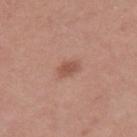The total-body-photography lesion software estimated an area of roughly 3.5 mm², an outline eccentricity of about 0.8 (0 = round, 1 = elongated), and two-axis asymmetry of about 0.2. The analysis additionally found roughly 9 lightness units darker than nearby skin and a normalized border contrast of about 7. And it measured a border-irregularity rating of about 2/10, a within-lesion color-variation index near 1.5/10, and a peripheral color-asymmetry measure near 0.5. It also reported a nevus-likeness score of about 85/100 and a lesion-detection confidence of about 100/100.
A female patient in their mid- to late 40s.
Cropped from a whole-body photographic skin survey; the tile spans about 15 mm.
Imaged with white-light lighting.
The lesion is on the leg.